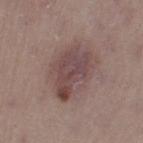The lesion was photographed on a routine skin check and not biopsied; there is no pathology result. On the left thigh. This image is a 15 mm lesion crop taken from a total-body photograph. Imaged with white-light lighting. The lesion's longest dimension is about 6 mm. Automated tile analysis of the lesion measured an area of roughly 14 mm². It also reported a normalized lesion–skin contrast near 8. And it measured a classifier nevus-likeness of about 15/100 and a lesion-detection confidence of about 100/100. A female patient approximately 50 years of age.